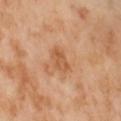A 15 mm close-up extracted from a 3D total-body photography capture.
An algorithmic analysis of the crop reported a footprint of about 4.5 mm², an outline eccentricity of about 0.75 (0 = round, 1 = elongated), and two-axis asymmetry of about 0.3. It also reported about 8 CIELAB-L* units darker than the surrounding skin and a normalized border contrast of about 6. The analysis additionally found a border-irregularity index near 3/10, a within-lesion color-variation index near 3/10, and peripheral color asymmetry of about 1.
On the abdomen.
The subject is a female aged 53 to 57.